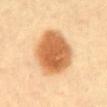{
  "biopsy_status": "not biopsied; imaged during a skin examination",
  "patient": {
    "age_approx": 60
  },
  "site": "abdomen",
  "image": {
    "source": "total-body photography crop",
    "field_of_view_mm": 15
  }
}